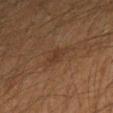– follow-up · catalogued during a skin exam; not biopsied
– subject · male, about 50 years old
– tile lighting · cross-polarized illumination
– image source · ~15 mm crop, total-body skin-cancer survey
– lesion diameter · ≈3.5 mm
– anatomic site · the left forearm